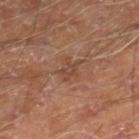Findings:
• site: the leg
• automated metrics: a footprint of about 4.5 mm² and two-axis asymmetry of about 0.4; about 6 CIELAB-L* units darker than the surrounding skin and a normalized border contrast of about 5.5; border irregularity of about 4.5 on a 0–10 scale, a within-lesion color-variation index near 2/10, and radial color variation of about 0.5; a classifier nevus-likeness of about 0/100 and a detector confidence of about 100 out of 100 that the crop contains a lesion
• subject: male, aged around 70
• image: ~15 mm crop, total-body skin-cancer survey
• size: ≈3 mm
• illumination: cross-polarized illumination